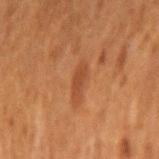imaging modality: ~15 mm crop, total-body skin-cancer survey; body site: the arm; subject: female, aged approximately 50.